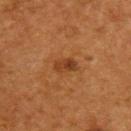Recorded during total-body skin imaging; not selected for excision or biopsy. Approximately 3 mm at its widest. The lesion is on the back. The patient is a male approximately 55 years of age. Cropped from a whole-body photographic skin survey; the tile spans about 15 mm. Automated tile analysis of the lesion measured a shape eccentricity near 0.85 and a symmetry-axis asymmetry near 0.2. Imaged with cross-polarized lighting.